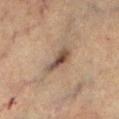Assessment:
Recorded during total-body skin imaging; not selected for excision or biopsy.
Image and clinical context:
The subject is a female aged 68 to 72. The lesion is on the leg. A 15 mm crop from a total-body photograph taken for skin-cancer surveillance. Automated image analysis of the tile measured a lesion area of about 6.5 mm² and a shape-asymmetry score of about 0.3 (0 = symmetric). And it measured a mean CIELAB color near L≈43 a*≈14 b*≈23, roughly 11 lightness units darker than nearby skin, and a normalized border contrast of about 9.